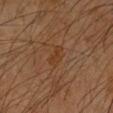A male patient, aged approximately 70. Cropped from a whole-body photographic skin survey; the tile spans about 15 mm. Measured at roughly 2.5 mm in maximum diameter. From the left forearm.